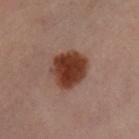Q: Was this lesion biopsied?
A: no biopsy performed (imaged during a skin exam)
Q: How was this image acquired?
A: ~15 mm tile from a whole-body skin photo
Q: What are the patient's age and sex?
A: female, aged around 60
Q: Where on the body is the lesion?
A: the leg